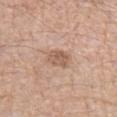This lesion was catalogued during total-body skin photography and was not selected for biopsy.
A male patient, in their 60s.
The total-body-photography lesion software estimated a lesion area of about 5 mm² and two-axis asymmetry of about 0.25. And it measured a border-irregularity index near 2/10 and a color-variation rating of about 3/10. The analysis additionally found a classifier nevus-likeness of about 15/100.
Longest diameter approximately 2.5 mm.
The lesion is on the left forearm.
A 15 mm close-up extracted from a 3D total-body photography capture.